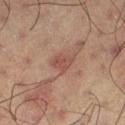biopsy status = no biopsy performed (imaged during a skin exam) | TBP lesion metrics = an eccentricity of roughly 0.65 and a symmetry-axis asymmetry near 0.25; a mean CIELAB color near L≈43 a*≈19 b*≈23, about 7 CIELAB-L* units darker than the surrounding skin, and a normalized border contrast of about 6; a border-irregularity index near 2/10, a within-lesion color-variation index near 3.5/10, and peripheral color asymmetry of about 1.5; an automated nevus-likeness rating near 15 out of 100 and lesion-presence confidence of about 100/100 | tile lighting = cross-polarized | patient = male, roughly 60 years of age | lesion diameter = about 3 mm | acquisition = ~15 mm tile from a whole-body skin photo.